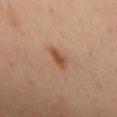| feature | finding |
|---|---|
| site | the upper back |
| imaging modality | ~15 mm crop, total-body skin-cancer survey |
| patient | male, aged around 55 |